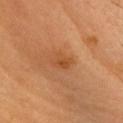Assessment: The lesion was tiled from a total-body skin photograph and was not biopsied. Acquisition and patient details: A male patient, aged around 60. From the head or neck. A close-up tile cropped from a whole-body skin photograph, about 15 mm across.